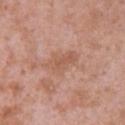Part of a total-body skin-imaging series; this lesion was reviewed on a skin check and was not flagged for biopsy.
A female patient, in their 40s.
A lesion tile, about 15 mm wide, cut from a 3D total-body photograph.
Measured at roughly 4 mm in maximum diameter.
The tile uses white-light illumination.
Located on the upper back.